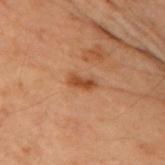From the left upper arm.
This is a cross-polarized tile.
A roughly 15 mm field-of-view crop from a total-body skin photograph.
A female subject aged 53–57.
Automated image analysis of the tile measured a mean CIELAB color near L≈40 a*≈22 b*≈32, roughly 9 lightness units darker than nearby skin, and a normalized lesion–skin contrast near 8. It also reported radial color variation of about 0.5.
Measured at roughly 3 mm in maximum diameter.